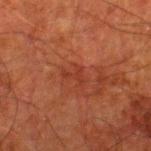Impression:
Imaged during a routine full-body skin examination; the lesion was not biopsied and no histopathology is available.
Image and clinical context:
Measured at roughly 3 mm in maximum diameter. The total-body-photography lesion software estimated a border-irregularity rating of about 7.5/10, a color-variation rating of about 0/10, and radial color variation of about 0. It also reported lesion-presence confidence of about 100/100. Imaged with cross-polarized lighting. The patient is a male about 80 years old. From the right lower leg. A region of skin cropped from a whole-body photographic capture, roughly 15 mm wide.